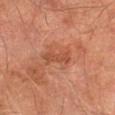| key | value |
|---|---|
| workup | no biopsy performed (imaged during a skin exam) |
| subject | male, aged 73–77 |
| site | the left thigh |
| acquisition | ~15 mm tile from a whole-body skin photo |